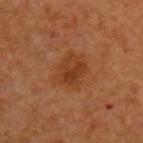biopsy_status: not biopsied; imaged during a skin examination
lighting: cross-polarized
patient:
  sex: male
  age_approx: 65
site: upper back
automated_metrics:
  area_mm2_approx: 12.0
  eccentricity: 0.6
  shape_asymmetry: 0.25
  border_irregularity_0_10: 3.0
  color_variation_0_10: 4.0
  peripheral_color_asymmetry: 1.5
  nevus_likeness_0_100: 30
  lesion_detection_confidence_0_100: 100
lesion_size:
  long_diameter_mm_approx: 4.5
image:
  source: total-body photography crop
  field_of_view_mm: 15